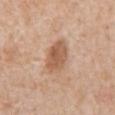The lesion was tiled from a total-body skin photograph and was not biopsied. A male subject, about 60 years old. Captured under white-light illumination. On the abdomen. Measured at roughly 4.5 mm in maximum diameter. The total-body-photography lesion software estimated an average lesion color of about L≈58 a*≈20 b*≈32 (CIELAB) and about 11 CIELAB-L* units darker than the surrounding skin. It also reported a nevus-likeness score of about 70/100 and a detector confidence of about 100 out of 100 that the crop contains a lesion. This image is a 15 mm lesion crop taken from a total-body photograph.